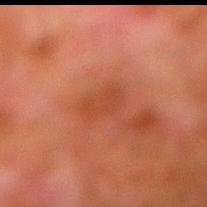Clinical impression: Imaged during a routine full-body skin examination; the lesion was not biopsied and no histopathology is available. Image and clinical context: A 15 mm close-up extracted from a 3D total-body photography capture. The lesion is located on the right lower leg. Captured under cross-polarized illumination. The patient is a male aged 78–82.